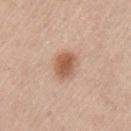Q: Who is the patient?
A: male, roughly 55 years of age
Q: What is the imaging modality?
A: total-body-photography crop, ~15 mm field of view
Q: Lesion size?
A: about 3.5 mm
Q: What is the anatomic site?
A: the arm
Q: What lighting was used for the tile?
A: white-light